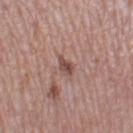Clinical impression:
Part of a total-body skin-imaging series; this lesion was reviewed on a skin check and was not flagged for biopsy.
Background:
Approximately 3 mm at its widest. A 15 mm crop from a total-body photograph taken for skin-cancer surveillance. A female patient, aged 38–42. On the left thigh.